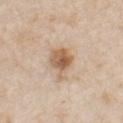Findings:
- biopsy status: imaged on a skin check; not biopsied
- lesion size: about 4 mm
- image source: ~15 mm tile from a whole-body skin photo
- subject: female, in their mid-40s
- body site: the front of the torso
- tile lighting: white-light illumination